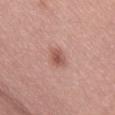Clinical summary:
Imaged with white-light lighting. A 15 mm crop from a total-body photograph taken for skin-cancer surveillance. On the back. The subject is a male aged 53–57.